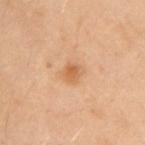| key | value |
|---|---|
| workup | imaged on a skin check; not biopsied |
| lesion diameter | ≈3 mm |
| body site | the left arm |
| subject | female, roughly 40 years of age |
| automated metrics | an eccentricity of roughly 0.6 and a symmetry-axis asymmetry near 0.25; a mean CIELAB color near L≈64 a*≈22 b*≈40, roughly 9 lightness units darker than nearby skin, and a normalized border contrast of about 6.5 |
| image source | total-body-photography crop, ~15 mm field of view |
| tile lighting | cross-polarized |